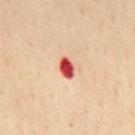Captured during whole-body skin photography for melanoma surveillance; the lesion was not biopsied.
The lesion is located on the chest.
Automated image analysis of the tile measured a footprint of about 4 mm², an outline eccentricity of about 0.8 (0 = round, 1 = elongated), and a symmetry-axis asymmetry near 0.25. And it measured a lesion color around L≈57 a*≈42 b*≈34 in CIELAB and a normalized lesion–skin contrast near 14. The analysis additionally found a nevus-likeness score of about 0/100 and lesion-presence confidence of about 100/100.
The tile uses cross-polarized illumination.
A male patient, aged approximately 55.
A 15 mm close-up extracted from a 3D total-body photography capture.